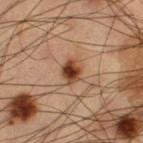Captured during whole-body skin photography for melanoma surveillance; the lesion was not biopsied. The lesion is located on the left thigh. The total-body-photography lesion software estimated an average lesion color of about L≈43 a*≈22 b*≈32 (CIELAB), a lesion–skin lightness drop of about 16, and a normalized lesion–skin contrast near 11.5. A male subject, approximately 60 years of age. The recorded lesion diameter is about 3 mm. Captured under cross-polarized illumination. A 15 mm close-up extracted from a 3D total-body photography capture.